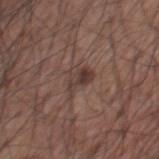workup: no biopsy performed (imaged during a skin exam)
location: the front of the torso
automated metrics: about 8 CIELAB-L* units darker than the surrounding skin and a normalized border contrast of about 7; an automated nevus-likeness rating near 35 out of 100 and lesion-presence confidence of about 100/100
lesion size: ≈3.5 mm
tile lighting: white-light illumination
acquisition: ~15 mm crop, total-body skin-cancer survey
patient: male, about 75 years old